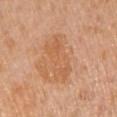biopsy status: total-body-photography surveillance lesion; no biopsy
image: total-body-photography crop, ~15 mm field of view
automated metrics: a lesion area of about 13 mm², a shape eccentricity near 0.9, and two-axis asymmetry of about 0.25; a mean CIELAB color near L≈60 a*≈23 b*≈38, about 7 CIELAB-L* units darker than the surrounding skin, and a lesion-to-skin contrast of about 5.5 (normalized; higher = more distinct)
location: the left upper arm
tile lighting: white-light illumination
lesion diameter: ≈6 mm
patient: female, roughly 55 years of age